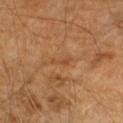Recorded during total-body skin imaging; not selected for excision or biopsy. On the arm. Captured under cross-polarized illumination. This image is a 15 mm lesion crop taken from a total-body photograph. A male subject aged 58 to 62.